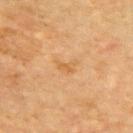Background: A female patient, in their 70s. Automated image analysis of the tile measured a classifier nevus-likeness of about 0/100 and a lesion-detection confidence of about 100/100. This is a cross-polarized tile. Cropped from a total-body skin-imaging series; the visible field is about 15 mm. Measured at roughly 2.5 mm in maximum diameter. Located on the back.